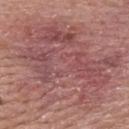Imaged during a routine full-body skin examination; the lesion was not biopsied and no histopathology is available.
About 13 mm across.
A male subject aged around 40.
This is a white-light tile.
A close-up tile cropped from a whole-body skin photograph, about 15 mm across.
On the back.
An algorithmic analysis of the crop reported a lesion-detection confidence of about 100/100.